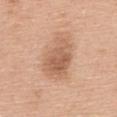Q: Is there a histopathology result?
A: total-body-photography surveillance lesion; no biopsy
Q: Lesion location?
A: the back
Q: How large is the lesion?
A: ≈6.5 mm
Q: How was this image acquired?
A: 15 mm crop, total-body photography
Q: Who is the patient?
A: female, roughly 60 years of age
Q: Automated lesion metrics?
A: a lesion–skin lightness drop of about 11